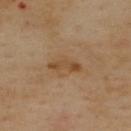The lesion was tiled from a total-body skin photograph and was not biopsied.
A lesion tile, about 15 mm wide, cut from a 3D total-body photograph.
The lesion is on the upper back.
The recorded lesion diameter is about 3.5 mm.
Imaged with cross-polarized lighting.
The subject is a male aged 53–57.